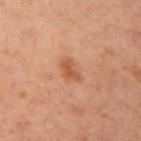The lesion was tiled from a total-body skin photograph and was not biopsied.
A lesion tile, about 15 mm wide, cut from a 3D total-body photograph.
The lesion is located on the left upper arm.
The total-body-photography lesion software estimated a normalized border contrast of about 7. And it measured a classifier nevus-likeness of about 40/100 and a lesion-detection confidence of about 100/100.
The tile uses cross-polarized illumination.
The patient is a female aged approximately 55.
Longest diameter approximately 3 mm.